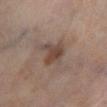Captured during whole-body skin photography for melanoma surveillance; the lesion was not biopsied. A male patient about 70 years old. This is a cross-polarized tile. A close-up tile cropped from a whole-body skin photograph, about 15 mm across. From the left lower leg. The total-body-photography lesion software estimated an area of roughly 7.5 mm², a shape eccentricity near 0.65, and a symmetry-axis asymmetry near 0.4. And it measured a lesion color around L≈42 a*≈15 b*≈23 in CIELAB, about 9 CIELAB-L* units darker than the surrounding skin, and a normalized lesion–skin contrast near 7.5. It also reported border irregularity of about 4.5 on a 0–10 scale, a color-variation rating of about 3/10, and a peripheral color-asymmetry measure near 1.